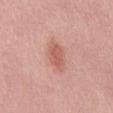Recorded during total-body skin imaging; not selected for excision or biopsy. From the mid back. A close-up tile cropped from a whole-body skin photograph, about 15 mm across. Automated tile analysis of the lesion measured a lesion–skin lightness drop of about 9 and a lesion-to-skin contrast of about 6.5 (normalized; higher = more distinct). The analysis additionally found a nevus-likeness score of about 70/100 and lesion-presence confidence of about 100/100. A male subject, aged around 30. Captured under white-light illumination. The lesion's longest dimension is about 3.5 mm.